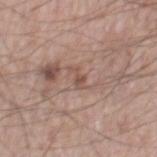Context: The lesion-visualizer software estimated an average lesion color of about L≈51 a*≈19 b*≈25 (CIELAB) and roughly 8 lightness units darker than nearby skin. And it measured border irregularity of about 6.5 on a 0–10 scale, a within-lesion color-variation index near 0/10, and peripheral color asymmetry of about 0. The analysis additionally found a classifier nevus-likeness of about 0/100 and lesion-presence confidence of about 90/100. A 15 mm close-up tile from a total-body photography series done for melanoma screening. A male subject about 60 years old. Located on the right thigh. The tile uses white-light illumination. About 2.5 mm across.